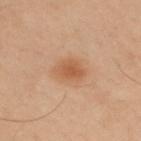diameter = about 3.5 mm
tile lighting = cross-polarized illumination
subject = male, aged 28 to 32
site = the upper back
acquisition = ~15 mm crop, total-body skin-cancer survey
automated lesion analysis = a color-variation rating of about 2.5/10; an automated nevus-likeness rating near 90 out of 100 and lesion-presence confidence of about 100/100